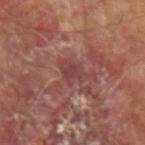| feature | finding |
|---|---|
| workup | no biopsy performed (imaged during a skin exam) |
| acquisition | total-body-photography crop, ~15 mm field of view |
| image-analysis metrics | an average lesion color of about L≈41 a*≈25 b*≈21 (CIELAB), a lesion–skin lightness drop of about 7, and a lesion-to-skin contrast of about 5.5 (normalized; higher = more distinct); a nevus-likeness score of about 0/100 |
| location | the left forearm |
| patient | male, aged approximately 65 |
| size | ≈3 mm |
| tile lighting | cross-polarized |